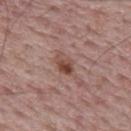The lesion was photographed on a routine skin check and not biopsied; there is no pathology result. About 2.5 mm across. A male patient, aged approximately 65. From the mid back. The total-body-photography lesion software estimated a footprint of about 4 mm², a shape eccentricity near 0.75, and a symmetry-axis asymmetry near 0.2. The software also gave a color-variation rating of about 4/10 and a peripheral color-asymmetry measure near 1. A 15 mm crop from a total-body photograph taken for skin-cancer surveillance.